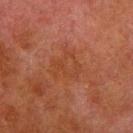The lesion was photographed on a routine skin check and not biopsied; there is no pathology result.
Automated tile analysis of the lesion measured a lesion color around L≈33 a*≈22 b*≈29 in CIELAB. And it measured an automated nevus-likeness rating near 0 out of 100.
Located on the right lower leg.
A 15 mm crop from a total-body photograph taken for skin-cancer surveillance.
Longest diameter approximately 4 mm.
The patient is a male aged approximately 80.